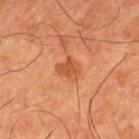  biopsy_status: not biopsied; imaged during a skin examination
  lighting: cross-polarized
  automated_metrics:
    border_irregularity_0_10: 3.0
    color_variation_0_10: 1.5
    peripheral_color_asymmetry: 0.5
    nevus_likeness_0_100: 50
    lesion_detection_confidence_0_100: 100
  image:
    source: total-body photography crop
    field_of_view_mm: 15
  patient:
    sex: male
    age_approx: 80
  site: left thigh
  lesion_size:
    long_diameter_mm_approx: 3.0